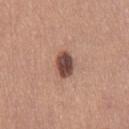The lesion was tiled from a total-body skin photograph and was not biopsied. A roughly 15 mm field-of-view crop from a total-body skin photograph. A female patient approximately 35 years of age. The lesion is on the right thigh. Measured at roughly 3.5 mm in maximum diameter. Automated image analysis of the tile measured an area of roughly 6.5 mm², an outline eccentricity of about 0.75 (0 = round, 1 = elongated), and a shape-asymmetry score of about 0.2 (0 = symmetric). The analysis additionally found a lesion color around L≈47 a*≈21 b*≈24 in CIELAB, roughly 17 lightness units darker than nearby skin, and a lesion-to-skin contrast of about 11.5 (normalized; higher = more distinct).